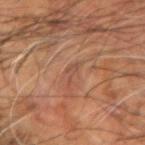follow-up: catalogued during a skin exam; not biopsied | patient: male, aged 58 to 62 | automated metrics: an area of roughly 2 mm²; about 6 CIELAB-L* units darker than the surrounding skin and a normalized border contrast of about 5; a border-irregularity rating of about 7/10, a within-lesion color-variation index near 0/10, and radial color variation of about 0; a classifier nevus-likeness of about 0/100 | body site: the left arm | illumination: cross-polarized illumination | image source: 15 mm crop, total-body photography | lesion diameter: ≈3 mm.